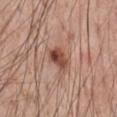Impression: Recorded during total-body skin imaging; not selected for excision or biopsy. Image and clinical context: The lesion is on the chest. A male subject, aged 53 to 57. A roughly 15 mm field-of-view crop from a total-body skin photograph.